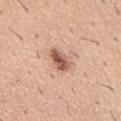Q: Is there a histopathology result?
A: no biopsy performed (imaged during a skin exam)
Q: Who is the patient?
A: male, in their 60s
Q: How was this image acquired?
A: ~15 mm tile from a whole-body skin photo
Q: Where on the body is the lesion?
A: the mid back
Q: Lesion size?
A: ≈3.5 mm